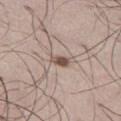{"biopsy_status": "not biopsied; imaged during a skin examination"}